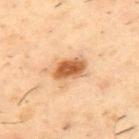Recorded during total-body skin imaging; not selected for excision or biopsy. A roughly 15 mm field-of-view crop from a total-body skin photograph. The lesion is located on the upper back. The subject is a male aged 53 to 57.